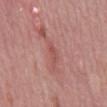• follow-up: total-body-photography surveillance lesion; no biopsy
• subject: male, aged approximately 50
• illumination: white-light illumination
• lesion diameter: ≈3 mm
• imaging modality: ~15 mm tile from a whole-body skin photo
• anatomic site: the mid back
• automated lesion analysis: border irregularity of about 3.5 on a 0–10 scale, a within-lesion color-variation index near 0/10, and peripheral color asymmetry of about 0